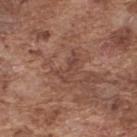Image and clinical context: From the upper back. The lesion-visualizer software estimated a footprint of about 5 mm² and two-axis asymmetry of about 0.45. Longest diameter approximately 3.5 mm. Cropped from a total-body skin-imaging series; the visible field is about 15 mm. A male patient, in their mid- to late 70s.